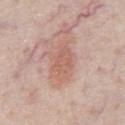workup: total-body-photography surveillance lesion; no biopsy | illumination: white-light illumination | subject: male, aged around 60 | anatomic site: the chest | image: 15 mm crop, total-body photography.